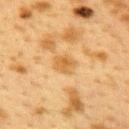The lesion was photographed on a routine skin check and not biopsied; there is no pathology result. The tile uses cross-polarized illumination. An algorithmic analysis of the crop reported about 8 CIELAB-L* units darker than the surrounding skin and a normalized border contrast of about 7. The analysis additionally found a border-irregularity rating of about 1.5/10, a color-variation rating of about 3/10, and radial color variation of about 1. The software also gave an automated nevus-likeness rating near 0 out of 100 and a detector confidence of about 100 out of 100 that the crop contains a lesion. About 3 mm across. A 15 mm crop from a total-body photograph taken for skin-cancer surveillance. A female subject aged approximately 40. Located on the upper back.